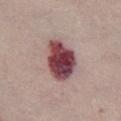Longest diameter approximately 5.5 mm.
The tile uses white-light illumination.
A female patient, aged around 65.
The lesion is on the front of the torso.
A region of skin cropped from a whole-body photographic capture, roughly 15 mm wide.